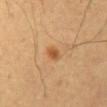  biopsy_status: not biopsied; imaged during a skin examination
  site: abdomen
  automated_metrics:
    border_irregularity_0_10: 2.0
    color_variation_0_10: 3.0
    peripheral_color_asymmetry: 1.0
    lesion_detection_confidence_0_100: 100
  image:
    source: total-body photography crop
    field_of_view_mm: 15
  lesion_size:
    long_diameter_mm_approx: 2.5
  lighting: cross-polarized
  patient:
    sex: male
    age_approx: 50